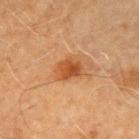notes = catalogued during a skin exam; not biopsied
lesion size = about 3 mm
image = ~15 mm crop, total-body skin-cancer survey
body site = the left upper arm
tile lighting = cross-polarized
subject = male, aged approximately 70
automated metrics = a mean CIELAB color near L≈42 a*≈23 b*≈35, about 10 CIELAB-L* units darker than the surrounding skin, and a normalized border contrast of about 8; a border-irregularity rating of about 2/10 and a peripheral color-asymmetry measure near 1.5; a detector confidence of about 100 out of 100 that the crop contains a lesion